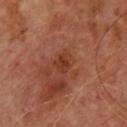Captured during whole-body skin photography for melanoma surveillance; the lesion was not biopsied.
The total-body-photography lesion software estimated a border-irregularity rating of about 2.5/10, a within-lesion color-variation index near 2/10, and peripheral color asymmetry of about 0.5.
This is a cross-polarized tile.
A 15 mm crop from a total-body photograph taken for skin-cancer surveillance.
A male subject approximately 65 years of age.
Approximately 2.5 mm at its widest.